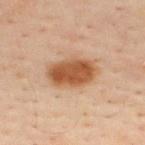| feature | finding |
|---|---|
| workup | total-body-photography surveillance lesion; no biopsy |